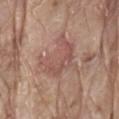No biopsy was performed on this lesion — it was imaged during a full skin examination and was not determined to be concerning. A male patient, aged 78–82. A 15 mm close-up tile from a total-body photography series done for melanoma screening. The lesion is on the lower back. The tile uses white-light illumination. Automated tile analysis of the lesion measured an area of roughly 14 mm² and a symmetry-axis asymmetry near 0.55. And it measured a lesion color around L≈53 a*≈21 b*≈24 in CIELAB. And it measured border irregularity of about 7.5 on a 0–10 scale, internal color variation of about 3 on a 0–10 scale, and radial color variation of about 1. The software also gave an automated nevus-likeness rating near 5 out of 100 and a detector confidence of about 100 out of 100 that the crop contains a lesion.